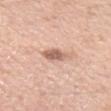Part of a total-body skin-imaging series; this lesion was reviewed on a skin check and was not flagged for biopsy. A 15 mm close-up tile from a total-body photography series done for melanoma screening. A female patient aged 38–42. On the left forearm.